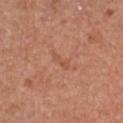Part of a total-body skin-imaging series; this lesion was reviewed on a skin check and was not flagged for biopsy.
A 15 mm close-up extracted from a 3D total-body photography capture.
A female patient in their 50s.
The total-body-photography lesion software estimated an area of roughly 2 mm², an eccentricity of roughly 0.95, and a shape-asymmetry score of about 0.4 (0 = symmetric). The analysis additionally found an average lesion color of about L≈53 a*≈25 b*≈33 (CIELAB) and a lesion-to-skin contrast of about 4.5 (normalized; higher = more distinct).
Longest diameter approximately 2.5 mm.
The tile uses white-light illumination.
From the chest.